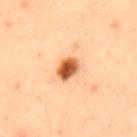A male subject in their mid- to late 30s. About 3 mm across. Automated tile analysis of the lesion measured a shape eccentricity near 0.7 and a symmetry-axis asymmetry near 0.15. A 15 mm crop from a total-body photograph taken for skin-cancer surveillance. The lesion is on the mid back. Imaged with cross-polarized lighting.